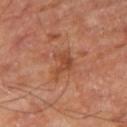Part of a total-body skin-imaging series; this lesion was reviewed on a skin check and was not flagged for biopsy. The subject is roughly 65 years of age. The lesion's longest dimension is about 4 mm. Located on the right thigh. The lesion-visualizer software estimated an area of roughly 6 mm², a shape eccentricity near 0.75, and a symmetry-axis asymmetry near 0.55. The software also gave border irregularity of about 6 on a 0–10 scale, a color-variation rating of about 2.5/10, and a peripheral color-asymmetry measure near 1. Captured under cross-polarized illumination. This image is a 15 mm lesion crop taken from a total-body photograph.